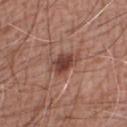Image and clinical context:
A male subject, approximately 60 years of age. Cropped from a whole-body photographic skin survey; the tile spans about 15 mm. Imaged with white-light lighting. Located on the arm.